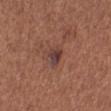Assessment: This lesion was catalogued during total-body skin photography and was not selected for biopsy. Acquisition and patient details: A female subject, roughly 55 years of age. A region of skin cropped from a whole-body photographic capture, roughly 15 mm wide. The tile uses white-light illumination. Located on the left lower leg. Measured at roughly 2.5 mm in maximum diameter.